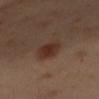The lesion was photographed on a routine skin check and not biopsied; there is no pathology result. This is a cross-polarized tile. A female patient aged around 40. The lesion's longest dimension is about 3.5 mm. From the left arm. A 15 mm close-up extracted from a 3D total-body photography capture.